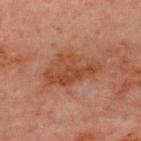tile lighting — cross-polarized illumination; site — the chest; patient — male, aged approximately 70; image source — ~15 mm tile from a whole-body skin photo; size — ~7 mm (longest diameter).